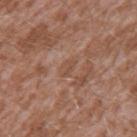patient = male, in their mid-40s
diameter = ~2.5 mm (longest diameter)
tile lighting = white-light illumination
location = the arm
acquisition = total-body-photography crop, ~15 mm field of view